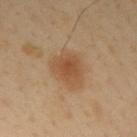Q: Was a biopsy performed?
A: imaged on a skin check; not biopsied
Q: Patient demographics?
A: male, aged 38 to 42
Q: Lesion location?
A: the right upper arm
Q: What kind of image is this?
A: ~15 mm tile from a whole-body skin photo